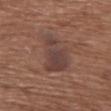Acquisition and patient details:
About 5.5 mm across. This image is a 15 mm lesion crop taken from a total-body photograph. The subject is a female in their mid- to late 70s. Located on the chest. Automated tile analysis of the lesion measured an area of roughly 14 mm², a shape eccentricity near 0.8, and a symmetry-axis asymmetry near 0.4. Imaged with white-light lighting.